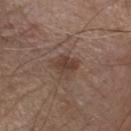Part of a total-body skin-imaging series; this lesion was reviewed on a skin check and was not flagged for biopsy.
The subject is a male roughly 80 years of age.
On the chest.
This image is a 15 mm lesion crop taken from a total-body photograph.
The recorded lesion diameter is about 3 mm.